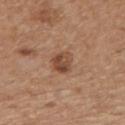Captured during whole-body skin photography for melanoma surveillance; the lesion was not biopsied.
Cropped from a total-body skin-imaging series; the visible field is about 15 mm.
A male subject, in their 70s.
From the mid back.